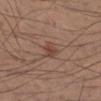subject = male, aged 33 to 37 | lesion diameter = ≈2.5 mm | anatomic site = the right lower leg | imaging modality = ~15 mm crop, total-body skin-cancer survey | automated metrics = a nevus-likeness score of about 25/100 and a detector confidence of about 100 out of 100 that the crop contains a lesion.